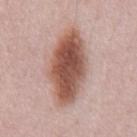Notes:
- biopsy status — no biopsy performed (imaged during a skin exam)
- site — the chest
- subject — male, aged 48 to 52
- imaging modality — total-body-photography crop, ~15 mm field of view
- tile lighting — white-light
- image-analysis metrics — an eccentricity of roughly 0.85 and a symmetry-axis asymmetry near 0.15
- size — ≈8.5 mm